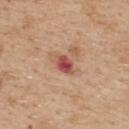Clinical impression:
Imaged during a routine full-body skin examination; the lesion was not biopsied and no histopathology is available.
Clinical summary:
Measured at roughly 3 mm in maximum diameter. On the upper back. A 15 mm close-up tile from a total-body photography series done for melanoma screening. This is a white-light tile. A female patient in their mid- to late 40s.